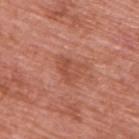workup = no biopsy performed (imaged during a skin exam) | size = ~3.5 mm (longest diameter) | tile lighting = white-light | subject = male, roughly 70 years of age | image = ~15 mm crop, total-body skin-cancer survey | site = the upper back.